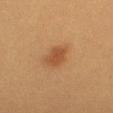The lesion is on the right thigh.
Imaged with cross-polarized lighting.
The patient is a female approximately 40 years of age.
A lesion tile, about 15 mm wide, cut from a 3D total-body photograph.